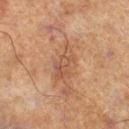Findings:
– workup — catalogued during a skin exam; not biopsied
– anatomic site — the right lower leg
– image — ~15 mm crop, total-body skin-cancer survey
– patient — male, aged around 70